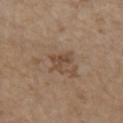Case summary:
- biopsy status — catalogued during a skin exam; not biopsied
- TBP lesion metrics — an automated nevus-likeness rating near 0 out of 100 and lesion-presence confidence of about 100/100
- image — ~15 mm crop, total-body skin-cancer survey
- site — the chest
- subject — female, roughly 65 years of age
- illumination — white-light
- diameter — about 3.5 mm